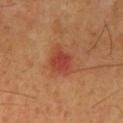Recorded during total-body skin imaging; not selected for excision or biopsy.
The lesion-visualizer software estimated a border-irregularity index near 3/10, internal color variation of about 3 on a 0–10 scale, and peripheral color asymmetry of about 1. The analysis additionally found lesion-presence confidence of about 100/100.
Imaged with cross-polarized lighting.
The patient is a male aged 58–62.
Cropped from a whole-body photographic skin survey; the tile spans about 15 mm.
Approximately 4 mm at its widest.
The lesion is located on the mid back.